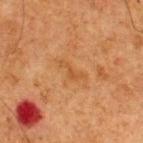follow-up: total-body-photography surveillance lesion; no biopsy | illumination: cross-polarized illumination | acquisition: total-body-photography crop, ~15 mm field of view | TBP lesion metrics: an area of roughly 3 mm², an eccentricity of roughly 0.9, and two-axis asymmetry of about 0.65; border irregularity of about 7 on a 0–10 scale, a within-lesion color-variation index near 0/10, and radial color variation of about 0 | subject: male, in their mid-60s | size: ~3.5 mm (longest diameter) | site: the upper back.